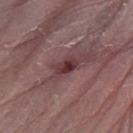biopsy_status: not biopsied; imaged during a skin examination
automated_metrics:
  area_mm2_approx: 3.5
  eccentricity: 0.85
  cielab_L: 34
  cielab_a: 24
  cielab_b: 16
  vs_skin_darker_L: 12.0
  vs_skin_contrast_norm: 10.5
site: right forearm
lesion_size:
  long_diameter_mm_approx: 2.5
lighting: white-light
image:
  source: total-body photography crop
  field_of_view_mm: 15
patient:
  sex: male
  age_approx: 65